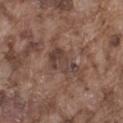biopsy_status: not biopsied; imaged during a skin examination
lesion_size:
  long_diameter_mm_approx: 4.0
patient:
  sex: male
  age_approx: 75
image:
  source: total-body photography crop
  field_of_view_mm: 15
site: left thigh